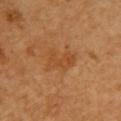follow-up: no biopsy performed (imaged during a skin exam)
lesion size: about 4 mm
patient: female, about 55 years old
site: the chest
acquisition: ~15 mm crop, total-body skin-cancer survey